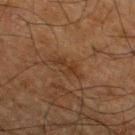Notes:
- follow-up · imaged on a skin check; not biopsied
- illumination · cross-polarized illumination
- lesion size · ~3.5 mm (longest diameter)
- anatomic site · the left lower leg
- automated lesion analysis · lesion-presence confidence of about 90/100
- subject · male, in their mid- to late 60s
- image source · ~15 mm tile from a whole-body skin photo